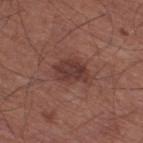Part of a total-body skin-imaging series; this lesion was reviewed on a skin check and was not flagged for biopsy.
A 15 mm crop from a total-body photograph taken for skin-cancer surveillance.
A male patient, approximately 65 years of age.
The lesion is located on the leg.
The lesion's longest dimension is about 4 mm.